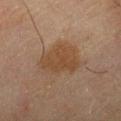notes: catalogued during a skin exam; not biopsied | location: the left thigh | subject: male, about 80 years old | imaging modality: ~15 mm tile from a whole-body skin photo | automated lesion analysis: a lesion color around L≈34 a*≈14 b*≈25 in CIELAB, a lesion–skin lightness drop of about 6, and a lesion-to-skin contrast of about 7 (normalized; higher = more distinct); a classifier nevus-likeness of about 20/100 and lesion-presence confidence of about 100/100 | lighting: cross-polarized.